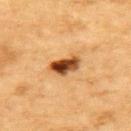workup — no biopsy performed (imaged during a skin exam) | automated metrics — a lesion area of about 6.5 mm², an eccentricity of roughly 0.8, and a shape-asymmetry score of about 0.2 (0 = symmetric); a lesion color around L≈42 a*≈23 b*≈37 in CIELAB, a lesion–skin lightness drop of about 20, and a normalized lesion–skin contrast near 14; a classifier nevus-likeness of about 100/100 and lesion-presence confidence of about 100/100 | body site — the upper back | patient — male, aged around 85 or older | lesion diameter — ≈3.5 mm | illumination — cross-polarized illumination | acquisition — ~15 mm tile from a whole-body skin photo.